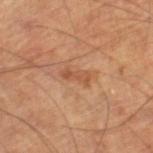Part of a total-body skin-imaging series; this lesion was reviewed on a skin check and was not flagged for biopsy.
On the right lower leg.
This is a cross-polarized tile.
An algorithmic analysis of the crop reported an average lesion color of about L≈51 a*≈23 b*≈34 (CIELAB) and a normalized lesion–skin contrast near 5.5. And it measured border irregularity of about 3 on a 0–10 scale, a color-variation rating of about 0.5/10, and peripheral color asymmetry of about 0.
A male patient about 60 years old.
Longest diameter approximately 3 mm.
A region of skin cropped from a whole-body photographic capture, roughly 15 mm wide.